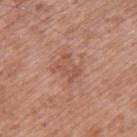Q: Is there a histopathology result?
A: no biopsy performed (imaged during a skin exam)
Q: What did automated image analysis measure?
A: a footprint of about 5.5 mm² and an outline eccentricity of about 0.75 (0 = round, 1 = elongated); an average lesion color of about L≈53 a*≈23 b*≈29 (CIELAB), a lesion–skin lightness drop of about 7, and a normalized border contrast of about 5
Q: What kind of image is this?
A: ~15 mm crop, total-body skin-cancer survey
Q: How large is the lesion?
A: ≈3 mm
Q: Patient demographics?
A: male, aged around 50
Q: Where on the body is the lesion?
A: the back